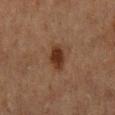Captured during whole-body skin photography for melanoma surveillance; the lesion was not biopsied.
The tile uses cross-polarized illumination.
Approximately 3.5 mm at its widest.
On the mid back.
Cropped from a total-body skin-imaging series; the visible field is about 15 mm.
A male patient aged 73 to 77.